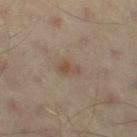Imaged during a routine full-body skin examination; the lesion was not biopsied and no histopathology is available. Imaged with cross-polarized lighting. Cropped from a total-body skin-imaging series; the visible field is about 15 mm. On the leg. The lesion's longest dimension is about 2.5 mm. A male subject aged approximately 55.